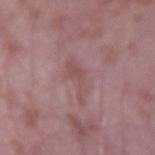Q: Was this lesion biopsied?
A: total-body-photography surveillance lesion; no biopsy
Q: What is the lesion's diameter?
A: about 4.5 mm
Q: What kind of image is this?
A: 15 mm crop, total-body photography
Q: What is the anatomic site?
A: the left upper arm
Q: What are the patient's age and sex?
A: male, in their mid- to late 60s
Q: What did automated image analysis measure?
A: an area of roughly 4.5 mm² and a shape eccentricity near 0.9; an average lesion color of about L≈50 a*≈21 b*≈19 (CIELAB) and a normalized border contrast of about 5.5